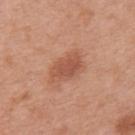A male subject approximately 70 years of age. Captured under white-light illumination. A region of skin cropped from a whole-body photographic capture, roughly 15 mm wide. The lesion is on the mid back. The total-body-photography lesion software estimated a mean CIELAB color near L≈53 a*≈25 b*≈32, about 10 CIELAB-L* units darker than the surrounding skin, and a normalized border contrast of about 6.5. Longest diameter approximately 4 mm.